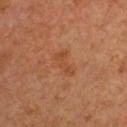follow-up: no biopsy performed (imaged during a skin exam); lighting: cross-polarized; subject: male, in their mid-60s; body site: the right upper arm; diameter: ~3.5 mm (longest diameter); image source: total-body-photography crop, ~15 mm field of view.